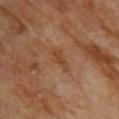This lesion was catalogued during total-body skin photography and was not selected for biopsy. A roughly 15 mm field-of-view crop from a total-body skin photograph. Approximately 3.5 mm at its widest. A female subject approximately 80 years of age. Automated tile analysis of the lesion measured an average lesion color of about L≈37 a*≈18 b*≈30 (CIELAB), roughly 5 lightness units darker than nearby skin, and a lesion-to-skin contrast of about 6 (normalized; higher = more distinct). The analysis additionally found a border-irregularity index near 3.5/10 and a within-lesion color-variation index near 1/10. The software also gave a lesion-detection confidence of about 100/100. From the back.